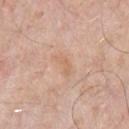Recorded during total-body skin imaging; not selected for excision or biopsy. A 15 mm crop from a total-body photograph taken for skin-cancer surveillance. Approximately 3 mm at its widest. An algorithmic analysis of the crop reported an average lesion color of about L≈64 a*≈20 b*≈33 (CIELAB), about 6 CIELAB-L* units darker than the surrounding skin, and a normalized lesion–skin contrast near 5. From the chest. The subject is a male aged around 70.